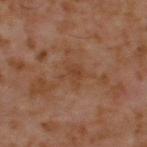Impression:
Recorded during total-body skin imaging; not selected for excision or biopsy.
Background:
Cropped from a whole-body photographic skin survey; the tile spans about 15 mm. A male subject in their 60s. The tile uses cross-polarized illumination. The lesion is located on the upper back.